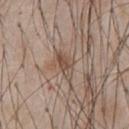Recorded during total-body skin imaging; not selected for excision or biopsy.
Measured at roughly 3.5 mm in maximum diameter.
Captured under white-light illumination.
A male subject in their 60s.
Automated image analysis of the tile measured a footprint of about 5.5 mm², an outline eccentricity of about 0.8 (0 = round, 1 = elongated), and two-axis asymmetry of about 0.35. And it measured a lesion–skin lightness drop of about 9 and a normalized lesion–skin contrast near 6.5. The software also gave an automated nevus-likeness rating near 0 out of 100 and a detector confidence of about 50 out of 100 that the crop contains a lesion.
A 15 mm close-up tile from a total-body photography series done for melanoma screening.
The lesion is located on the abdomen.